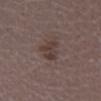<tbp_lesion>
  <biopsy_status>not biopsied; imaged during a skin examination</biopsy_status>
  <image>
    <source>total-body photography crop</source>
    <field_of_view_mm>15</field_of_view_mm>
  </image>
  <lesion_size>
    <long_diameter_mm_approx>3.5</long_diameter_mm_approx>
  </lesion_size>
  <lighting>white-light</lighting>
  <patient>
    <sex>female</sex>
    <age_approx>30</age_approx>
  </patient>
  <site>right lower leg</site>
  <automated_metrics>
    <area_mm2_approx>5.5</area_mm2_approx>
    <shape_asymmetry>0.4</shape_asymmetry>
    <cielab_L>37</cielab_L>
    <cielab_a>14</cielab_a>
    <cielab_b>19</cielab_b>
    <vs_skin_darker_L>7.0</vs_skin_darker_L>
    <vs_skin_contrast_norm>7.0</vs_skin_contrast_norm>
    <border_irregularity_0_10>4.5</border_irregularity_0_10>
    <color_variation_0_10>3.0</color_variation_0_10>
    <peripheral_color_asymmetry>1.0</peripheral_color_asymmetry>
  </automated_metrics>
</tbp_lesion>